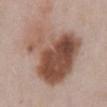  biopsy_status: not biopsied; imaged during a skin examination
  lighting: white-light
  automated_metrics:
    area_mm2_approx: 48.0
    shape_asymmetry: 0.45
    cielab_L: 51
    cielab_a: 19
    cielab_b: 27
    vs_skin_darker_L: 15.0
    color_variation_0_10: 10.0
    peripheral_color_asymmetry: 4.0
    nevus_likeness_0_100: 55
    lesion_detection_confidence_0_100: 100
  site: chest
  patient:
    sex: male
    age_approx: 55
  lesion_size:
    long_diameter_mm_approx: 9.5
  image:
    source: total-body photography crop
    field_of_view_mm: 15